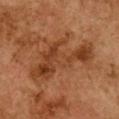* notes · catalogued during a skin exam; not biopsied
* site · the front of the torso
* subject · female, aged 58 to 62
* image · ~15 mm crop, total-body skin-cancer survey